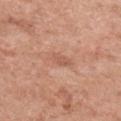Impression: The lesion was tiled from a total-body skin photograph and was not biopsied. Context: On the chest. The patient is a female approximately 50 years of age. A 15 mm close-up tile from a total-body photography series done for melanoma screening. Measured at roughly 3.5 mm in maximum diameter. Captured under white-light illumination.